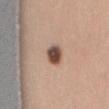Located on the mid back. Longest diameter approximately 3 mm. Captured under white-light illumination. This image is a 15 mm lesion crop taken from a total-body photograph. A female patient, aged around 25.